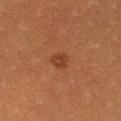This lesion was catalogued during total-body skin photography and was not selected for biopsy. The lesion is on the left thigh. A 15 mm close-up tile from a total-body photography series done for melanoma screening. The patient is a female aged around 30.